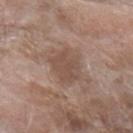| feature | finding |
|---|---|
| biopsy status | no biopsy performed (imaged during a skin exam) |
| illumination | white-light illumination |
| site | the left forearm |
| patient | female, in their mid- to late 70s |
| image source | 15 mm crop, total-body photography |